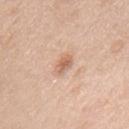Clinical impression:
Captured during whole-body skin photography for melanoma surveillance; the lesion was not biopsied.
Image and clinical context:
The lesion is located on the back. The lesion's longest dimension is about 2.5 mm. A male subject aged around 65. Automated tile analysis of the lesion measured a lesion color around L≈63 a*≈21 b*≈32 in CIELAB, about 11 CIELAB-L* units darker than the surrounding skin, and a lesion-to-skin contrast of about 7 (normalized; higher = more distinct). The analysis additionally found an automated nevus-likeness rating near 50 out of 100 and a detector confidence of about 100 out of 100 that the crop contains a lesion. A 15 mm crop from a total-body photograph taken for skin-cancer surveillance. Captured under white-light illumination.